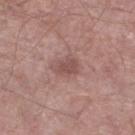biopsy_status: not biopsied; imaged during a skin examination
site: left lower leg
automated_metrics:
  area_mm2_approx: 5.0
  shape_asymmetry: 0.2
  border_irregularity_0_10: 2.0
  color_variation_0_10: 2.0
  peripheral_color_asymmetry: 1.0
  nevus_likeness_0_100: 10
  lesion_detection_confidence_0_100: 100
lesion_size:
  long_diameter_mm_approx: 3.0
image:
  source: total-body photography crop
  field_of_view_mm: 15
lighting: white-light
patient:
  sex: male
  age_approx: 55